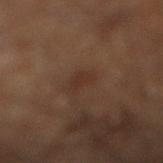Q: Was this lesion biopsied?
A: no biopsy performed (imaged during a skin exam)
Q: How large is the lesion?
A: ~3 mm (longest diameter)
Q: Where on the body is the lesion?
A: the right lower leg
Q: How was this image acquired?
A: total-body-photography crop, ~15 mm field of view
Q: Patient demographics?
A: male, in their 60s
Q: How was the tile lit?
A: cross-polarized illumination